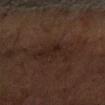Notes:
– notes · total-body-photography surveillance lesion; no biopsy
– location · the left forearm
– lighting · cross-polarized
– patient · male, approximately 60 years of age
– image · ~15 mm crop, total-body skin-cancer survey
– lesion size · ≈5 mm